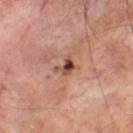Q: Was a biopsy performed?
A: catalogued during a skin exam; not biopsied
Q: What is the imaging modality?
A: ~15 mm crop, total-body skin-cancer survey
Q: Lesion location?
A: the left thigh
Q: What are the patient's age and sex?
A: male, approximately 70 years of age
Q: Lesion size?
A: ~2.5 mm (longest diameter)
Q: Illumination type?
A: cross-polarized